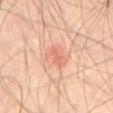notes — no biopsy performed (imaged during a skin exam) | diameter — ≈2.5 mm | patient — male, aged approximately 70 | anatomic site — the abdomen | automated metrics — a lesion area of about 2 mm², an eccentricity of roughly 0.95, and a shape-asymmetry score of about 0.4 (0 = symmetric); a color-variation rating of about 0/10 and a peripheral color-asymmetry measure near 0 | imaging modality — ~15 mm crop, total-body skin-cancer survey | tile lighting — cross-polarized illumination.